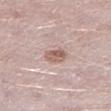Case summary:
– follow-up · imaged on a skin check; not biopsied
– imaging modality · ~15 mm tile from a whole-body skin photo
– illumination · white-light
– subject · male, about 50 years old
– lesion size · ≈2.5 mm
– site · the left lower leg